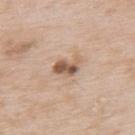  biopsy_status: not biopsied; imaged during a skin examination
  site: upper back
  automated_metrics:
    vs_skin_darker_L: 14.0
    vs_skin_contrast_norm: 9.5
  lesion_size:
    long_diameter_mm_approx: 3.0
  patient:
    sex: male
    age_approx: 65
  lighting: white-light
  image:
    source: total-body photography crop
    field_of_view_mm: 15